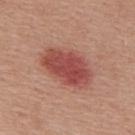Q: Was a biopsy performed?
A: imaged on a skin check; not biopsied
Q: Automated lesion metrics?
A: a footprint of about 18 mm², a shape eccentricity near 0.8, and two-axis asymmetry of about 0.15; a normalized border contrast of about 9
Q: Lesion size?
A: ~6.5 mm (longest diameter)
Q: What are the patient's age and sex?
A: male, roughly 55 years of age
Q: How was this image acquired?
A: 15 mm crop, total-body photography
Q: How was the tile lit?
A: white-light
Q: What is the anatomic site?
A: the back